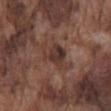Notes:
• follow-up — catalogued during a skin exam; not biopsied
• patient — male, about 75 years old
• image source — ~15 mm tile from a whole-body skin photo
• TBP lesion metrics — a lesion area of about 9 mm² and two-axis asymmetry of about 0.4; roughly 9 lightness units darker than nearby skin and a normalized lesion–skin contrast near 8; a border-irregularity rating of about 4.5/10, internal color variation of about 5 on a 0–10 scale, and a peripheral color-asymmetry measure near 1.5
• tile lighting — white-light illumination
• lesion diameter — ≈5 mm
• anatomic site — the back